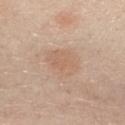Clinical impression:
The lesion was photographed on a routine skin check and not biopsied; there is no pathology result.
Clinical summary:
Longest diameter approximately 4.5 mm. A female subject, aged 38 to 42. Automated image analysis of the tile measured a lesion area of about 12 mm² and two-axis asymmetry of about 0.2. The software also gave a within-lesion color-variation index near 2.5/10 and a peripheral color-asymmetry measure near 1. It also reported an automated nevus-likeness rating near 5 out of 100 and a lesion-detection confidence of about 100/100. This is a white-light tile. The lesion is on the front of the torso. Cropped from a total-body skin-imaging series; the visible field is about 15 mm.